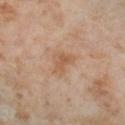Notes:
- follow-up — total-body-photography surveillance lesion; no biopsy
- lesion size — ≈3 mm
- site — the left thigh
- image — ~15 mm tile from a whole-body skin photo
- patient — female, aged 53 to 57